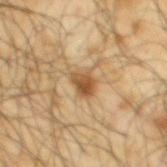{"biopsy_status": "not biopsied; imaged during a skin examination", "site": "upper back", "lesion_size": {"long_diameter_mm_approx": 2.5}, "image": {"source": "total-body photography crop", "field_of_view_mm": 15}, "patient": {"sex": "male", "age_approx": 65}}